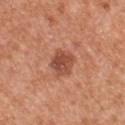Findings:
* follow-up: no biopsy performed (imaged during a skin exam)
* size: about 3 mm
* image source: total-body-photography crop, ~15 mm field of view
* automated lesion analysis: a border-irregularity rating of about 2/10, a within-lesion color-variation index near 3.5/10, and radial color variation of about 1; a classifier nevus-likeness of about 65/100 and a lesion-detection confidence of about 100/100
* site: the chest
* illumination: white-light illumination
* patient: male, aged 53 to 57